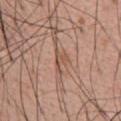Assessment:
Recorded during total-body skin imaging; not selected for excision or biopsy.
Context:
A 15 mm close-up extracted from a 3D total-body photography capture. A male patient, approximately 55 years of age. Located on the abdomen. This is a white-light tile. The lesion's longest dimension is about 2.5 mm.